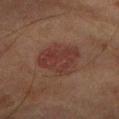follow-up = catalogued during a skin exam; not biopsied
site = the right lower leg
subject = male, in their mid-80s or older
automated metrics = an average lesion color of about L≈30 a*≈19 b*≈20 (CIELAB), roughly 6 lightness units darker than nearby skin, and a normalized lesion–skin contrast near 7; lesion-presence confidence of about 100/100
lesion size = about 5.5 mm
tile lighting = cross-polarized illumination
imaging modality = ~15 mm crop, total-body skin-cancer survey